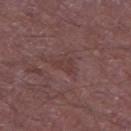The lesion was tiled from a total-body skin photograph and was not biopsied.
The lesion is on the leg.
Approximately 2.5 mm at its widest.
The patient is a male aged approximately 65.
Cropped from a whole-body photographic skin survey; the tile spans about 15 mm.